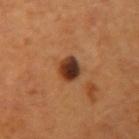A male patient in their mid-50s.
This image is a 15 mm lesion crop taken from a total-body photograph.
Imaged with cross-polarized lighting.
From the arm.
The lesion's longest dimension is about 3 mm.